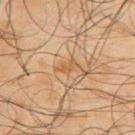This lesion was catalogued during total-body skin photography and was not selected for biopsy.